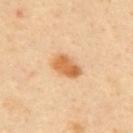Captured during whole-body skin photography for melanoma surveillance; the lesion was not biopsied. The tile uses cross-polarized illumination. The total-body-photography lesion software estimated a lesion–skin lightness drop of about 13 and a lesion-to-skin contrast of about 9 (normalized; higher = more distinct). And it measured a nevus-likeness score of about 100/100 and a detector confidence of about 100 out of 100 that the crop contains a lesion. The patient is a male about 40 years old. The lesion is located on the mid back. Approximately 3.5 mm at its widest. This image is a 15 mm lesion crop taken from a total-body photograph.